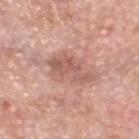notes — total-body-photography surveillance lesion; no biopsy